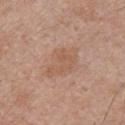Captured during whole-body skin photography for melanoma surveillance; the lesion was not biopsied. The lesion is on the front of the torso. The lesion's longest dimension is about 5 mm. Cropped from a total-body skin-imaging series; the visible field is about 15 mm. A male patient aged approximately 30.